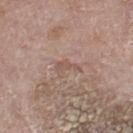This lesion was catalogued during total-body skin photography and was not selected for biopsy. Automated image analysis of the tile measured a mean CIELAB color near L≈53 a*≈17 b*≈25 and a lesion-to-skin contrast of about 5 (normalized; higher = more distinct). The analysis additionally found a border-irregularity rating of about 5.5/10. Located on the left thigh. A lesion tile, about 15 mm wide, cut from a 3D total-body photograph. The patient is a male roughly 75 years of age. Measured at roughly 2.5 mm in maximum diameter. Imaged with white-light lighting.